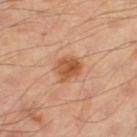follow-up: no biopsy performed (imaged during a skin exam)
location: the left thigh
patient: male, in their mid-50s
lighting: cross-polarized
image-analysis metrics: a shape-asymmetry score of about 0.3 (0 = symmetric)
lesion diameter: ≈3 mm
image source: total-body-photography crop, ~15 mm field of view